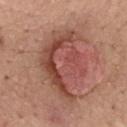Recorded during total-body skin imaging; not selected for excision or biopsy.
This is a white-light tile.
The lesion is located on the mid back.
A male subject, in their 50s.
Automated image analysis of the tile measured a lesion area of about 34 mm² and an outline eccentricity of about 0.75 (0 = round, 1 = elongated). And it measured border irregularity of about 3.5 on a 0–10 scale, a color-variation rating of about 8.5/10, and peripheral color asymmetry of about 3. The software also gave an automated nevus-likeness rating near 25 out of 100 and a lesion-detection confidence of about 90/100.
Longest diameter approximately 8.5 mm.
A 15 mm crop from a total-body photograph taken for skin-cancer surveillance.